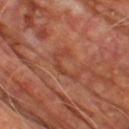Notes:
* workup · imaged on a skin check; not biopsied
* site · the upper back
* lesion diameter · ≈4.5 mm
* image · ~15 mm tile from a whole-body skin photo
* patient · male, aged approximately 60
* automated metrics · an area of roughly 4.5 mm² and a symmetry-axis asymmetry near 0.75; a lesion color around L≈41 a*≈27 b*≈31 in CIELAB
* lighting · cross-polarized